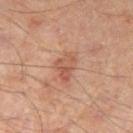Assessment: Recorded during total-body skin imaging; not selected for excision or biopsy. Context: The tile uses cross-polarized illumination. A roughly 15 mm field-of-view crop from a total-body skin photograph. The lesion is located on the right thigh. The patient is a male aged 63 to 67.